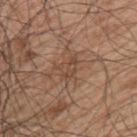Part of a total-body skin-imaging series; this lesion was reviewed on a skin check and was not flagged for biopsy. The lesion's longest dimension is about 3 mm. The subject is a male aged 48 to 52. Automated image analysis of the tile measured a classifier nevus-likeness of about 0/100 and a detector confidence of about 90 out of 100 that the crop contains a lesion. Imaged with white-light lighting. From the back. Cropped from a total-body skin-imaging series; the visible field is about 15 mm.